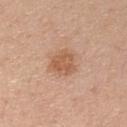Image and clinical context: On the chest. This is a white-light tile. Automated image analysis of the tile measured an area of roughly 7 mm² and two-axis asymmetry of about 0.3. The software also gave an average lesion color of about L≈58 a*≈22 b*≈34 (CIELAB) and roughly 9 lightness units darker than nearby skin. A male patient, roughly 40 years of age. A 15 mm close-up tile from a total-body photography series done for melanoma screening. Measured at roughly 3 mm in maximum diameter.